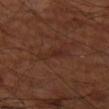| feature | finding |
|---|---|
| biopsy status | imaged on a skin check; not biopsied |
| image | ~15 mm crop, total-body skin-cancer survey |
| location | the left thigh |
| subject | male, about 70 years old |
| size | ≈4 mm |
| tile lighting | cross-polarized illumination |